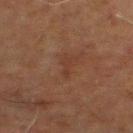follow-up=no biopsy performed (imaged during a skin exam)
lesion diameter=about 2.5 mm
anatomic site=the right lower leg
lighting=cross-polarized illumination
image-analysis metrics=border irregularity of about 5 on a 0–10 scale, a color-variation rating of about 0/10, and a peripheral color-asymmetry measure near 0
image source=15 mm crop, total-body photography
patient=male, about 70 years old